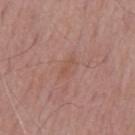Assessment:
Imaged during a routine full-body skin examination; the lesion was not biopsied and no histopathology is available.
Acquisition and patient details:
The subject is a male roughly 65 years of age. The lesion's longest dimension is about 2.5 mm. The tile uses white-light illumination. The lesion is located on the mid back. Cropped from a whole-body photographic skin survey; the tile spans about 15 mm.